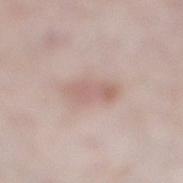Q: What are the patient's age and sex?
A: male, in their 40s
Q: What is the anatomic site?
A: the right lower leg
Q: How large is the lesion?
A: ≈4 mm
Q: How was this image acquired?
A: 15 mm crop, total-body photography
Q: What did automated image analysis measure?
A: a lesion color around L≈62 a*≈18 b*≈23 in CIELAB, roughly 8 lightness units darker than nearby skin, and a normalized border contrast of about 5.5; a border-irregularity rating of about 2.5/10 and internal color variation of about 2.5 on a 0–10 scale; an automated nevus-likeness rating near 20 out of 100 and a lesion-detection confidence of about 100/100
Q: Illumination type?
A: white-light illumination